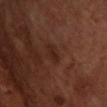Imaged during a routine full-body skin examination; the lesion was not biopsied and no histopathology is available. Longest diameter approximately 3 mm. This image is a 15 mm lesion crop taken from a total-body photograph. From the chest. The patient is a male about 70 years old.